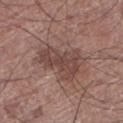<tbp_lesion>
<biopsy_status>not biopsied; imaged during a skin examination</biopsy_status>
<site>left lower leg</site>
<image>
  <source>total-body photography crop</source>
  <field_of_view_mm>15</field_of_view_mm>
</image>
<lighting>white-light</lighting>
<patient>
  <sex>male</sex>
  <age_approx>75</age_approx>
</patient>
<lesion_size>
  <long_diameter_mm_approx>5.5</long_diameter_mm_approx>
</lesion_size>
</tbp_lesion>